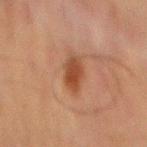* workup — total-body-photography surveillance lesion; no biopsy
* site — the abdomen
* subject — male, aged 63–67
* image source — 15 mm crop, total-body photography
* lesion size — ~3.5 mm (longest diameter)